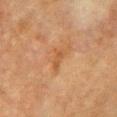{"biopsy_status": "not biopsied; imaged during a skin examination", "lighting": "cross-polarized", "site": "chest", "image": {"source": "total-body photography crop", "field_of_view_mm": 15}, "patient": {"sex": "female", "age_approx": 55}}